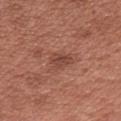Q: Was a biopsy performed?
A: imaged on a skin check; not biopsied
Q: Patient demographics?
A: male, in their mid-20s
Q: Lesion size?
A: ≈3 mm
Q: How was the tile lit?
A: white-light illumination
Q: Lesion location?
A: the chest
Q: What did automated image analysis measure?
A: a footprint of about 5 mm², an outline eccentricity of about 0.75 (0 = round, 1 = elongated), and a symmetry-axis asymmetry near 0.35; an average lesion color of about L≈45 a*≈24 b*≈28 (CIELAB) and a lesion–skin lightness drop of about 8
Q: What kind of image is this?
A: total-body-photography crop, ~15 mm field of view